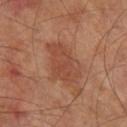Assessment: The lesion was tiled from a total-body skin photograph and was not biopsied. Background: The lesion is on the right lower leg. A lesion tile, about 15 mm wide, cut from a 3D total-body photograph. Automated image analysis of the tile measured an area of roughly 13 mm² and an outline eccentricity of about 0.8 (0 = round, 1 = elongated). The analysis additionally found border irregularity of about 3.5 on a 0–10 scale and a peripheral color-asymmetry measure near 1. The subject is a male about 70 years old. Captured under cross-polarized illumination.